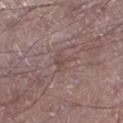  biopsy_status: not biopsied; imaged during a skin examination
  image:
    source: total-body photography crop
    field_of_view_mm: 15
  patient:
    sex: male
    age_approx: 50
  lesion_size:
    long_diameter_mm_approx: 2.5
  site: left lower leg
  lighting: white-light